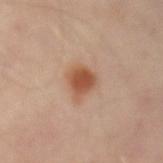Imaged during a routine full-body skin examination; the lesion was not biopsied and no histopathology is available.
Cropped from a total-body skin-imaging series; the visible field is about 15 mm.
A patient aged around 55.
The lesion is located on the left forearm.
About 3.5 mm across.
This is a cross-polarized tile.
Automated image analysis of the tile measured a border-irregularity index near 2.5/10, internal color variation of about 3 on a 0–10 scale, and a peripheral color-asymmetry measure near 0.5. And it measured a nevus-likeness score of about 100/100 and a lesion-detection confidence of about 100/100.